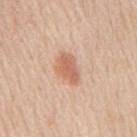Assessment: Imaged during a routine full-body skin examination; the lesion was not biopsied and no histopathology is available.